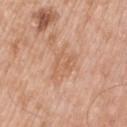Imaged during a routine full-body skin examination; the lesion was not biopsied and no histopathology is available.
A close-up tile cropped from a whole-body skin photograph, about 15 mm across.
On the left upper arm.
Imaged with white-light lighting.
A male patient, aged around 50.